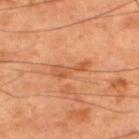follow-up = no biopsy performed (imaged during a skin exam) | tile lighting = cross-polarized | lesion size = ≈4.5 mm | patient = male, about 60 years old | automated lesion analysis = a mean CIELAB color near L≈55 a*≈27 b*≈39 and a normalized lesion–skin contrast near 6; border irregularity of about 5.5 on a 0–10 scale, internal color variation of about 2 on a 0–10 scale, and peripheral color asymmetry of about 0.5; a nevus-likeness score of about 0/100 and a detector confidence of about 100 out of 100 that the crop contains a lesion | anatomic site = the upper back | imaging modality = ~15 mm tile from a whole-body skin photo.